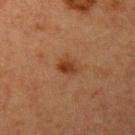Part of a total-body skin-imaging series; this lesion was reviewed on a skin check and was not flagged for biopsy.
This image is a 15 mm lesion crop taken from a total-body photograph.
Measured at roughly 2.5 mm in maximum diameter.
The lesion is on the left upper arm.
A female subject roughly 40 years of age.
Captured under cross-polarized illumination.